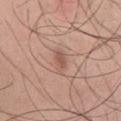follow-up: total-body-photography surveillance lesion; no biopsy | image: total-body-photography crop, ~15 mm field of view | size: ≈3 mm | anatomic site: the chest | automated lesion analysis: a lesion area of about 3 mm², an eccentricity of roughly 0.9, and a shape-asymmetry score of about 0.35 (0 = symmetric); internal color variation of about 0.5 on a 0–10 scale and radial color variation of about 0 | patient: male, aged 43 to 47 | lighting: white-light.